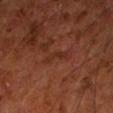Notes:
* notes · total-body-photography surveillance lesion; no biopsy
* imaging modality · ~15 mm crop, total-body skin-cancer survey
* location · the left forearm
* subject · male, about 60 years old